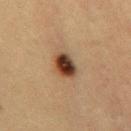Automated image analysis of the tile measured a footprint of about 7.5 mm² and a symmetry-axis asymmetry near 0.15. The software also gave a mean CIELAB color near L≈34 a*≈16 b*≈26, a lesion–skin lightness drop of about 15, and a normalized border contrast of about 13.
The tile uses cross-polarized illumination.
The patient is a female about 40 years old.
A 15 mm close-up extracted from a 3D total-body photography capture.
The lesion is located on the mid back.
Measured at roughly 3.5 mm in maximum diameter.